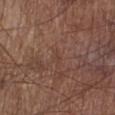Clinical impression: Imaged during a routine full-body skin examination; the lesion was not biopsied and no histopathology is available. Clinical summary: The lesion is located on the right lower leg. The patient is a male roughly 55 years of age. This image is a 15 mm lesion crop taken from a total-body photograph. Imaged with white-light lighting. Measured at roughly 1 mm in maximum diameter. The lesion-visualizer software estimated a mean CIELAB color near L≈40 a*≈20 b*≈25 and roughly 4 lightness units darker than nearby skin. And it measured a border-irregularity index near 2.5/10 and a color-variation rating of about 0/10.